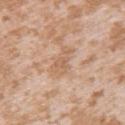| key | value |
|---|---|
| notes | no biopsy performed (imaged during a skin exam) |
| lighting | white-light illumination |
| image source | 15 mm crop, total-body photography |
| subject | female, aged 23 to 27 |
| location | the arm |